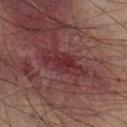<case>
  <biopsy_status>not biopsied; imaged during a skin examination</biopsy_status>
  <site>right lower leg</site>
  <lighting>cross-polarized</lighting>
  <image>
    <source>total-body photography crop</source>
    <field_of_view_mm>15</field_of_view_mm>
  </image>
  <lesion_size>
    <long_diameter_mm_approx>5.5</long_diameter_mm_approx>
  </lesion_size>
  <patient>
    <sex>male</sex>
    <age_approx>75</age_approx>
  </patient>
</case>